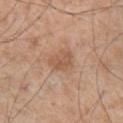A region of skin cropped from a whole-body photographic capture, roughly 15 mm wide. The subject is a male aged 53 to 57. On the chest.